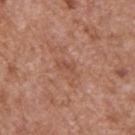A male patient, in their mid- to late 60s.
The lesion is on the back.
A lesion tile, about 15 mm wide, cut from a 3D total-body photograph.